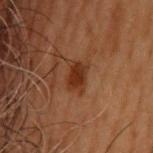– notes — total-body-photography surveillance lesion; no biopsy
– patient — male, aged 53 to 57
– diameter — ~3 mm (longest diameter)
– location — the upper back
– image source — ~15 mm crop, total-body skin-cancer survey
– TBP lesion metrics — an area of roughly 6.5 mm² and a shape eccentricity near 0.75; an average lesion color of about L≈27 a*≈20 b*≈27 (CIELAB) and a normalized lesion–skin contrast near 8.5; a border-irregularity index near 2/10, a color-variation rating of about 2.5/10, and a peripheral color-asymmetry measure near 1; lesion-presence confidence of about 100/100